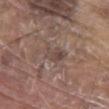Assessment: Captured during whole-body skin photography for melanoma surveillance; the lesion was not biopsied. Clinical summary: An algorithmic analysis of the crop reported a mean CIELAB color near L≈45 a*≈15 b*≈21, about 7 CIELAB-L* units darker than the surrounding skin, and a normalized lesion–skin contrast near 6. It also reported a nevus-likeness score of about 0/100 and lesion-presence confidence of about 70/100. Measured at roughly 3 mm in maximum diameter. Cropped from a total-body skin-imaging series; the visible field is about 15 mm. Imaged with white-light lighting. The subject is a male aged 78–82. On the abdomen.